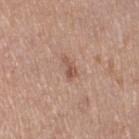Imaged during a routine full-body skin examination; the lesion was not biopsied and no histopathology is available. A 15 mm close-up extracted from a 3D total-body photography capture. Imaged with white-light lighting. The lesion is on the right thigh. Longest diameter approximately 2.5 mm. A female subject, in their 40s. Automated tile analysis of the lesion measured a lesion area of about 2.5 mm², an eccentricity of roughly 0.9, and a symmetry-axis asymmetry near 0.5.